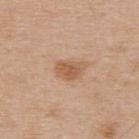{"biopsy_status": "not biopsied; imaged during a skin examination", "site": "upper back", "lighting": "white-light", "automated_metrics": {"cielab_L": 57, "cielab_a": 20, "cielab_b": 34, "vs_skin_darker_L": 10.0, "vs_skin_contrast_norm": 7.0, "border_irregularity_0_10": 2.0, "peripheral_color_asymmetry": 0.5}, "lesion_size": {"long_diameter_mm_approx": 3.0}, "image": {"source": "total-body photography crop", "field_of_view_mm": 15}, "patient": {"sex": "male", "age_approx": 70}}